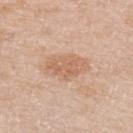– illumination · white-light illumination
– patient · female, aged 43 to 47
– anatomic site · the left upper arm
– lesion size · about 5 mm
– image · ~15 mm tile from a whole-body skin photo
– automated metrics · border irregularity of about 2.5 on a 0–10 scale, a color-variation rating of about 3/10, and peripheral color asymmetry of about 1